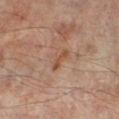Captured during whole-body skin photography for melanoma surveillance; the lesion was not biopsied. A male subject, aged approximately 65. Located on the right lower leg. Measured at roughly 3 mm in maximum diameter. Imaged with cross-polarized lighting. A roughly 15 mm field-of-view crop from a total-body skin photograph.